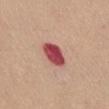The lesion was tiled from a total-body skin photograph and was not biopsied.
Located on the abdomen.
Captured under white-light illumination.
A female patient, aged approximately 50.
A 15 mm close-up extracted from a 3D total-body photography capture.
Automated image analysis of the tile measured an area of roughly 7.5 mm², a shape eccentricity near 0.7, and a shape-asymmetry score of about 0.2 (0 = symmetric). The analysis additionally found a border-irregularity index near 1.5/10, internal color variation of about 4.5 on a 0–10 scale, and a peripheral color-asymmetry measure near 1.5.